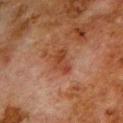workup: imaged on a skin check; not biopsied
illumination: cross-polarized
imaging modality: ~15 mm crop, total-body skin-cancer survey
lesion diameter: about 3.5 mm
patient: male, about 80 years old
location: the upper back